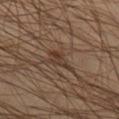  biopsy_status: not biopsied; imaged during a skin examination
  image:
    source: total-body photography crop
    field_of_view_mm: 15
  patient:
    sex: male
    age_approx: 45
  lesion_size:
    long_diameter_mm_approx: 3.0
  site: leg